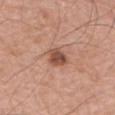notes — total-body-photography surveillance lesion; no biopsy
automated metrics — a lesion area of about 5.5 mm², an eccentricity of roughly 0.55, and a symmetry-axis asymmetry near 0.25; a lesion color around L≈51 a*≈23 b*≈29 in CIELAB, about 13 CIELAB-L* units darker than the surrounding skin, and a normalized border contrast of about 9; border irregularity of about 2.5 on a 0–10 scale and a peripheral color-asymmetry measure near 1; a nevus-likeness score of about 55/100 and lesion-presence confidence of about 100/100
subject — male, in their mid-70s
acquisition — 15 mm crop, total-body photography
location — the left upper arm
lesion diameter — about 2.5 mm
lighting — white-light illumination